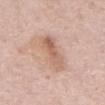* notes: catalogued during a skin exam; not biopsied
* lesion size: about 5 mm
* image-analysis metrics: roughly 10 lightness units darker than nearby skin
* imaging modality: ~15 mm tile from a whole-body skin photo
* location: the abdomen
* patient: male, aged around 70